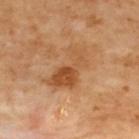body site: the back | image-analysis metrics: a lesion color around L≈54 a*≈24 b*≈40 in CIELAB, a lesion–skin lightness drop of about 9, and a lesion-to-skin contrast of about 7 (normalized; higher = more distinct) | lighting: cross-polarized illumination | acquisition: ~15 mm crop, total-body skin-cancer survey | lesion diameter: about 6 mm.